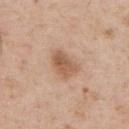| feature | finding |
|---|---|
| notes | total-body-photography surveillance lesion; no biopsy |
| size | ≈4 mm |
| subject | male, approximately 55 years of age |
| image source | ~15 mm tile from a whole-body skin photo |
| tile lighting | white-light |
| location | the left upper arm |